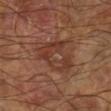Imaged during a routine full-body skin examination; the lesion was not biopsied and no histopathology is available. On the left lower leg. The patient is a male aged 58–62. A lesion tile, about 15 mm wide, cut from a 3D total-body photograph.